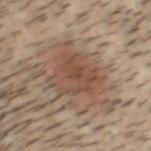This lesion was catalogued during total-body skin photography and was not selected for biopsy.
The subject is a male aged 28 to 32.
The tile uses cross-polarized illumination.
The lesion is on the head or neck.
The recorded lesion diameter is about 6 mm.
This image is a 15 mm lesion crop taken from a total-body photograph.